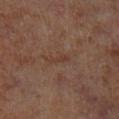Findings:
- follow-up — catalogued during a skin exam; not biopsied
- acquisition — ~15 mm crop, total-body skin-cancer survey
- patient — male, approximately 70 years of age
- body site — the left lower leg